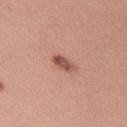The lesion was tiled from a total-body skin photograph and was not biopsied.
The tile uses white-light illumination.
A 15 mm crop from a total-body photograph taken for skin-cancer surveillance.
The patient is a female about 35 years old.
The lesion is located on the right upper arm.